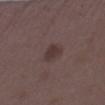Q: Lesion location?
A: the right lower leg
Q: Who is the patient?
A: female, aged 33–37
Q: What is the lesion's diameter?
A: about 3 mm
Q: What kind of image is this?
A: ~15 mm tile from a whole-body skin photo